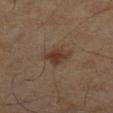Findings:
- follow-up · no biopsy performed (imaged during a skin exam)
- image source · ~15 mm crop, total-body skin-cancer survey
- patient · male, aged around 60
- anatomic site · the right lower leg
- size · about 2.5 mm
- lighting · cross-polarized
- automated metrics · an average lesion color of about L≈31 a*≈15 b*≈24 (CIELAB), a lesion–skin lightness drop of about 8, and a normalized lesion–skin contrast near 8; a border-irregularity index near 2.5/10, internal color variation of about 2.5 on a 0–10 scale, and radial color variation of about 1; a nevus-likeness score of about 70/100